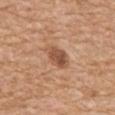workup: total-body-photography surveillance lesion; no biopsy
anatomic site: the upper back
automated metrics: a border-irregularity index near 2/10, a within-lesion color-variation index near 4/10, and radial color variation of about 1.5; a classifier nevus-likeness of about 40/100 and lesion-presence confidence of about 100/100
size: ~3.5 mm (longest diameter)
patient: female, aged 68 to 72
imaging modality: ~15 mm crop, total-body skin-cancer survey
illumination: white-light illumination